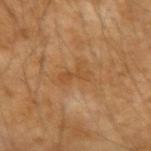Q: Was a biopsy performed?
A: no biopsy performed (imaged during a skin exam)
Q: What is the lesion's diameter?
A: ≈3.5 mm
Q: How was the tile lit?
A: cross-polarized illumination
Q: Who is the patient?
A: male, aged 53 to 57
Q: What is the imaging modality?
A: ~15 mm tile from a whole-body skin photo
Q: What is the anatomic site?
A: the left forearm
Q: Automated lesion metrics?
A: a mean CIELAB color near L≈46 a*≈20 b*≈36, roughly 6 lightness units darker than nearby skin, and a lesion-to-skin contrast of about 5.5 (normalized; higher = more distinct); a lesion-detection confidence of about 100/100